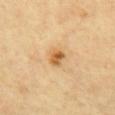Q: What kind of image is this?
A: total-body-photography crop, ~15 mm field of view
Q: What is the lesion's diameter?
A: ~2.5 mm (longest diameter)
Q: Lesion location?
A: the front of the torso
Q: Who is the patient?
A: aged around 60